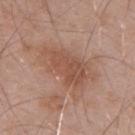biopsy_status: not biopsied; imaged during a skin examination
lesion_size:
  long_diameter_mm_approx: 7.0
patient:
  sex: male
  age_approx: 55
site: mid back
image:
  source: total-body photography crop
  field_of_view_mm: 15
lighting: white-light